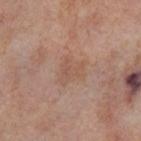illumination: cross-polarized; location: the right thigh; lesion diameter: ~3.5 mm (longest diameter); imaging modality: ~15 mm tile from a whole-body skin photo; subject: female, in their mid-50s.